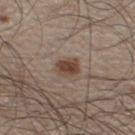  biopsy_status: not biopsied; imaged during a skin examination
  image:
    source: total-body photography crop
    field_of_view_mm: 15
  automated_metrics:
    vs_skin_contrast_norm: 9.0
  patient:
    sex: male
    age_approx: 60
  lighting: white-light
  site: left lower leg
  lesion_size:
    long_diameter_mm_approx: 3.0